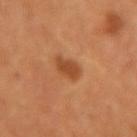Captured during whole-body skin photography for melanoma surveillance; the lesion was not biopsied. The lesion is located on the left forearm. Longest diameter approximately 3 mm. A female patient aged around 35. A 15 mm crop from a total-body photograph taken for skin-cancer surveillance.